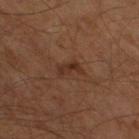biopsy_status: not biopsied; imaged during a skin examination
lesion_size:
  long_diameter_mm_approx: 3.0
patient:
  sex: male
  age_approx: 60
site: right thigh
image:
  source: total-body photography crop
  field_of_view_mm: 15